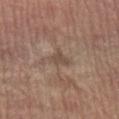workup = imaged on a skin check; not biopsied
site = the right lower leg
patient = female, aged 63–67
diameter = ~2.5 mm (longest diameter)
lighting = white-light
acquisition = ~15 mm tile from a whole-body skin photo